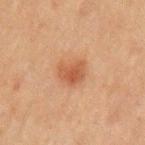Notes:
• follow-up — total-body-photography surveillance lesion; no biopsy
• lesion size — ~3 mm (longest diameter)
• location — the left upper arm
• subject — male, aged approximately 50
• tile lighting — cross-polarized illumination
• image-analysis metrics — a classifier nevus-likeness of about 90/100
• image — ~15 mm crop, total-body skin-cancer survey